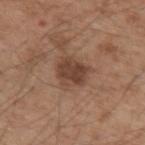The lesion was photographed on a routine skin check and not biopsied; there is no pathology result.
From the arm.
A male patient in their mid- to late 50s.
Captured under white-light illumination.
Longest diameter approximately 4 mm.
A 15 mm crop from a total-body photograph taken for skin-cancer surveillance.